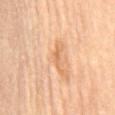{"biopsy_status": "not biopsied; imaged during a skin examination", "lighting": "cross-polarized", "automated_metrics": {"area_mm2_approx": 2.5, "eccentricity": 0.9, "shape_asymmetry": 0.45, "color_variation_0_10": 0.0, "peripheral_color_asymmetry": 0.0, "lesion_detection_confidence_0_100": 70}, "patient": {"sex": "female", "age_approx": 65}, "lesion_size": {"long_diameter_mm_approx": 2.5}, "site": "mid back", "image": {"source": "total-body photography crop", "field_of_view_mm": 15}}